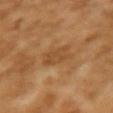image source = ~15 mm tile from a whole-body skin photo | lesion diameter = about 3 mm | tile lighting = cross-polarized | automated metrics = a lesion area of about 6 mm² and two-axis asymmetry of about 0.25; an automated nevus-likeness rating near 0 out of 100 and a detector confidence of about 100 out of 100 that the crop contains a lesion | subject = female, aged approximately 70 | body site = the right forearm.